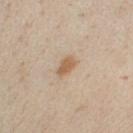workup — no biopsy performed (imaged during a skin exam)
anatomic site — the chest
patient — male, in their 30s
image source — total-body-photography crop, ~15 mm field of view